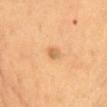Acquisition and patient details: The lesion is on the chest. A roughly 15 mm field-of-view crop from a total-body skin photograph. A female patient, about 65 years old.